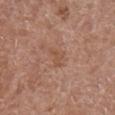Case summary:
– patient · male, aged approximately 75
– illumination · white-light illumination
– lesion size · ~2.5 mm (longest diameter)
– image · ~15 mm crop, total-body skin-cancer survey
– body site · the leg